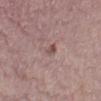Q: Was this lesion biopsied?
A: imaged on a skin check; not biopsied
Q: Patient demographics?
A: female, aged 48–52
Q: Lesion location?
A: the left lower leg
Q: How was this image acquired?
A: total-body-photography crop, ~15 mm field of view